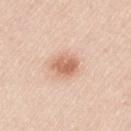Q: What kind of image is this?
A: ~15 mm tile from a whole-body skin photo
Q: What lighting was used for the tile?
A: white-light
Q: Lesion size?
A: ≈3.5 mm
Q: Where on the body is the lesion?
A: the left upper arm
Q: What are the patient's age and sex?
A: male, aged approximately 45